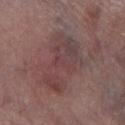Clinical impression: No biopsy was performed on this lesion — it was imaged during a full skin examination and was not determined to be concerning. Context: The lesion's longest dimension is about 7 mm. A female subject, roughly 65 years of age. This is a white-light tile. From the left thigh. This image is a 15 mm lesion crop taken from a total-body photograph.